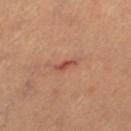Clinical impression: No biopsy was performed on this lesion — it was imaged during a full skin examination and was not determined to be concerning. Acquisition and patient details: The lesion-visualizer software estimated an outline eccentricity of about 0.95 (0 = round, 1 = elongated) and a shape-asymmetry score of about 0.4 (0 = symmetric). The subject is a female about 45 years old. Cropped from a total-body skin-imaging series; the visible field is about 15 mm. The lesion is on the leg. The lesion's longest dimension is about 2.5 mm.